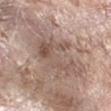Q: Is there a histopathology result?
A: catalogued during a skin exam; not biopsied
Q: Who is the patient?
A: female, approximately 75 years of age
Q: What is the imaging modality?
A: ~15 mm crop, total-body skin-cancer survey
Q: Lesion size?
A: ~6 mm (longest diameter)
Q: What lighting was used for the tile?
A: white-light illumination
Q: What is the anatomic site?
A: the right forearm